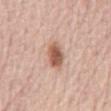notes: no biopsy performed (imaged during a skin exam); subject: female, aged approximately 65; body site: the abdomen; image: ~15 mm tile from a whole-body skin photo.